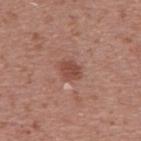{"biopsy_status": "not biopsied; imaged during a skin examination", "lighting": "white-light", "site": "mid back", "patient": {"sex": "male", "age_approx": 70}, "image": {"source": "total-body photography crop", "field_of_view_mm": 15}, "lesion_size": {"long_diameter_mm_approx": 2.5}, "automated_metrics": {"area_mm2_approx": 4.0, "eccentricity": 0.65, "shape_asymmetry": 0.25, "vs_skin_darker_L": 10.0, "vs_skin_contrast_norm": 7.5, "border_irregularity_0_10": 2.0, "color_variation_0_10": 2.0, "peripheral_color_asymmetry": 0.5, "lesion_detection_confidence_0_100": 100}}